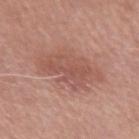Assessment: Imaged during a routine full-body skin examination; the lesion was not biopsied and no histopathology is available. Background: The lesion-visualizer software estimated an average lesion color of about L≈53 a*≈23 b*≈26 (CIELAB), about 8 CIELAB-L* units darker than the surrounding skin, and a normalized lesion–skin contrast near 5.5. And it measured a border-irregularity rating of about 4.5/10, a within-lesion color-variation index near 3/10, and peripheral color asymmetry of about 1. From the right upper arm. Measured at roughly 6.5 mm in maximum diameter. A close-up tile cropped from a whole-body skin photograph, about 15 mm across. The subject is a female aged 48 to 52.